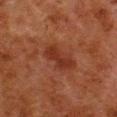follow-up — catalogued during a skin exam; not biopsied | lighting — cross-polarized | lesion size — ≈4 mm | automated metrics — a color-variation rating of about 2/10 and peripheral color asymmetry of about 0.5; a classifier nevus-likeness of about 35/100 | imaging modality — ~15 mm crop, total-body skin-cancer survey | subject — male, about 80 years old | anatomic site — the right lower leg.